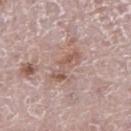workup=catalogued during a skin exam; not biopsied | body site=the left leg | imaging modality=~15 mm crop, total-body skin-cancer survey | patient=male, aged around 70.